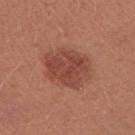notes: no biopsy performed (imaged during a skin exam); patient: female, in their mid-20s; body site: the left upper arm; image source: total-body-photography crop, ~15 mm field of view.